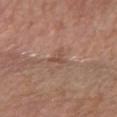Assessment: Captured during whole-body skin photography for melanoma surveillance; the lesion was not biopsied. Clinical summary: The lesion is on the right forearm. About 2.5 mm across. The lesion-visualizer software estimated an average lesion color of about L≈50 a*≈20 b*≈26 (CIELAB) and a normalized border contrast of about 5.5. The software also gave an automated nevus-likeness rating near 0 out of 100 and a lesion-detection confidence of about 90/100. This is a white-light tile. A 15 mm crop from a total-body photograph taken for skin-cancer surveillance. A female subject, in their mid-50s.